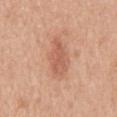notes = catalogued during a skin exam; not biopsied
patient = female, aged 38 to 42
anatomic site = the mid back
acquisition = 15 mm crop, total-body photography
tile lighting = white-light illumination